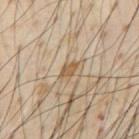Case summary:
• notes — catalogued during a skin exam; not biopsied
• image source — total-body-photography crop, ~15 mm field of view
• tile lighting — cross-polarized illumination
• patient — male, roughly 55 years of age
• size — ~2.5 mm (longest diameter)
• location — the chest